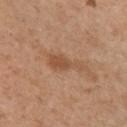Q: Is there a histopathology result?
A: imaged on a skin check; not biopsied
Q: Automated lesion metrics?
A: a lesion area of about 6 mm², an eccentricity of roughly 0.9, and a shape-asymmetry score of about 0.5 (0 = symmetric); a mean CIELAB color near L≈51 a*≈21 b*≈33 and a normalized border contrast of about 6
Q: Where on the body is the lesion?
A: the arm
Q: How large is the lesion?
A: ~4.5 mm (longest diameter)
Q: What is the imaging modality?
A: 15 mm crop, total-body photography
Q: Who is the patient?
A: female, roughly 30 years of age